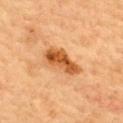The lesion was tiled from a total-body skin photograph and was not biopsied. On the back. This is a cross-polarized tile. A female subject roughly 60 years of age. Measured at roughly 5 mm in maximum diameter. Cropped from a total-body skin-imaging series; the visible field is about 15 mm.